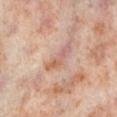Notes:
- biopsy status: imaged on a skin check; not biopsied
- image: ~15 mm crop, total-body skin-cancer survey
- subject: female, approximately 50 years of age
- body site: the right lower leg